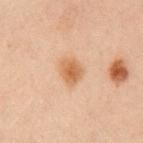biopsy status: total-body-photography surveillance lesion; no biopsy
site: the arm
patient: female, aged approximately 30
acquisition: total-body-photography crop, ~15 mm field of view